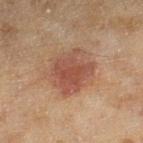Clinical impression: The lesion was photographed on a routine skin check and not biopsied; there is no pathology result. Acquisition and patient details: A female patient aged 58 to 62. From the leg. Approximately 5 mm at its widest. Cropped from a whole-body photographic skin survey; the tile spans about 15 mm. The lesion-visualizer software estimated an automated nevus-likeness rating near 90 out of 100 and a lesion-detection confidence of about 100/100.